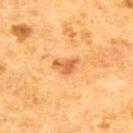<case>
  <site>upper back</site>
  <image>
    <source>total-body photography crop</source>
    <field_of_view_mm>15</field_of_view_mm>
  </image>
  <lesion_size>
    <long_diameter_mm_approx>3.0</long_diameter_mm_approx>
  </lesion_size>
  <automated_metrics>
    <area_mm2_approx>4.0</area_mm2_approx>
    <eccentricity>0.75</eccentricity>
    <shape_asymmetry>0.5</shape_asymmetry>
    <peripheral_color_asymmetry>1.0</peripheral_color_asymmetry>
  </automated_metrics>
  <patient>
    <sex>male</sex>
    <age_approx>50</age_approx>
  </patient>
  <lighting>cross-polarized</lighting>
</case>